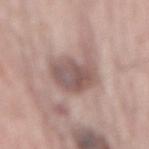| field | value |
|---|---|
| follow-up | imaged on a skin check; not biopsied |
| patient | male, about 65 years old |
| site | the mid back |
| automated metrics | a nevus-likeness score of about 50/100 and a lesion-detection confidence of about 100/100 |
| image | ~15 mm tile from a whole-body skin photo |
| lesion diameter | about 5.5 mm |